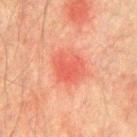Impression:
The lesion was photographed on a routine skin check and not biopsied; there is no pathology result.
Image and clinical context:
The subject is a male about 75 years old. The lesion is on the chest. Cropped from a total-body skin-imaging series; the visible field is about 15 mm.